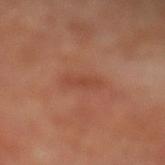Impression: No biopsy was performed on this lesion — it was imaged during a full skin examination and was not determined to be concerning. Image and clinical context: This is a cross-polarized tile. A male patient, about 65 years old. A 15 mm close-up extracted from a 3D total-body photography capture. The lesion's longest dimension is about 3.5 mm. The lesion is located on the leg.